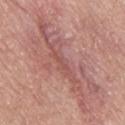Part of a total-body skin-imaging series; this lesion was reviewed on a skin check and was not flagged for biopsy. From the abdomen. A 15 mm crop from a total-body photograph taken for skin-cancer surveillance. Longest diameter approximately 14.5 mm. A male patient aged 53 to 57.